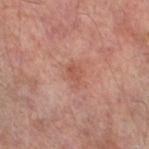Recorded during total-body skin imaging; not selected for excision or biopsy. The lesion is on the left thigh. A male patient aged 63–67. A close-up tile cropped from a whole-body skin photograph, about 15 mm across.